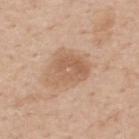<record>
  <biopsy_status>not biopsied; imaged during a skin examination</biopsy_status>
  <patient>
    <sex>male</sex>
    <age_approx>60</age_approx>
  </patient>
  <image>
    <source>total-body photography crop</source>
    <field_of_view_mm>15</field_of_view_mm>
  </image>
  <site>back</site>
  <automated_metrics>
    <area_mm2_approx>12.0</area_mm2_approx>
    <eccentricity>0.65</eccentricity>
    <shape_asymmetry>0.3</shape_asymmetry>
    <cielab_L>59</cielab_L>
    <cielab_a>19</cielab_a>
    <cielab_b>32</cielab_b>
    <vs_skin_darker_L>9.0</vs_skin_darker_L>
    <vs_skin_contrast_norm>6.0</vs_skin_contrast_norm>
    <border_irregularity_0_10>3.5</border_irregularity_0_10>
    <color_variation_0_10>3.5</color_variation_0_10>
    <peripheral_color_asymmetry>1.0</peripheral_color_asymmetry>
  </automated_metrics>
  <lighting>white-light</lighting>
</record>